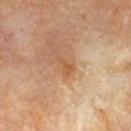Assessment:
Imaged during a routine full-body skin examination; the lesion was not biopsied and no histopathology is available.
Acquisition and patient details:
From the chest. A male subject roughly 60 years of age. A 15 mm close-up extracted from a 3D total-body photography capture. Imaged with cross-polarized lighting.